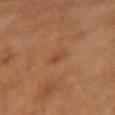Part of a total-body skin-imaging series; this lesion was reviewed on a skin check and was not flagged for biopsy. Approximately 1.5 mm at its widest. Cropped from a total-body skin-imaging series; the visible field is about 15 mm. The lesion-visualizer software estimated an area of roughly 1.5 mm², a shape eccentricity near 0.4, and a symmetry-axis asymmetry near 0.25. And it measured internal color variation of about 0 on a 0–10 scale and a peripheral color-asymmetry measure near 0. The lesion is on the right forearm. The patient is a female about 50 years old.